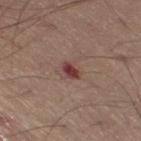Part of a total-body skin-imaging series; this lesion was reviewed on a skin check and was not flagged for biopsy. Captured under white-light illumination. Cropped from a whole-body photographic skin survey; the tile spans about 15 mm. Measured at roughly 2.5 mm in maximum diameter. The lesion is located on the right thigh. The subject is a male aged 53–57.